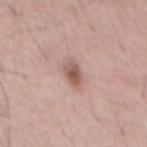The lesion was tiled from a total-body skin photograph and was not biopsied.
A male subject, in their mid- to late 50s.
Captured under white-light illumination.
Cropped from a whole-body photographic skin survey; the tile spans about 15 mm.